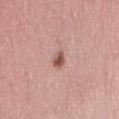Imaged during a routine full-body skin examination; the lesion was not biopsied and no histopathology is available. A close-up tile cropped from a whole-body skin photograph, about 15 mm across. Captured under white-light illumination. The patient is a female in their 50s. From the right thigh.